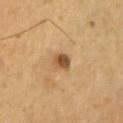{
  "biopsy_status": "not biopsied; imaged during a skin examination",
  "automated_metrics": {
    "color_variation_0_10": 4.5,
    "peripheral_color_asymmetry": 1.5
  },
  "lesion_size": {
    "long_diameter_mm_approx": 2.5
  },
  "site": "chest",
  "image": {
    "source": "total-body photography crop",
    "field_of_view_mm": 15
  },
  "patient": {
    "sex": "male",
    "age_approx": 45
  }
}